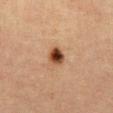workup: total-body-photography surveillance lesion; no biopsy
image source: 15 mm crop, total-body photography
subject: female, aged 38 to 42
diameter: ≈2.5 mm
site: the abdomen